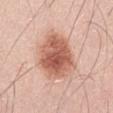- workup · catalogued during a skin exam; not biopsied
- illumination · white-light
- image · ~15 mm crop, total-body skin-cancer survey
- lesion diameter · ~6.5 mm (longest diameter)
- subject · male, aged approximately 50
- anatomic site · the right thigh